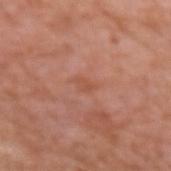Clinical impression:
This lesion was catalogued during total-body skin photography and was not selected for biopsy.
Acquisition and patient details:
Located on the left forearm. The patient is a male aged 73–77. This image is a 15 mm lesion crop taken from a total-body photograph. Approximately 2.5 mm at its widest. This is a white-light tile.